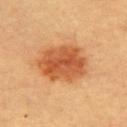Impression: Recorded during total-body skin imaging; not selected for excision or biopsy. Image and clinical context: This image is a 15 mm lesion crop taken from a total-body photograph. A female subject, roughly 60 years of age. The lesion's longest dimension is about 6 mm. Imaged with cross-polarized lighting. The lesion is on the upper back.